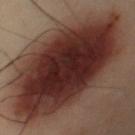The lesion is on the chest. A region of skin cropped from a whole-body photographic capture, roughly 15 mm wide. A male subject aged around 50. Longest diameter approximately 17.5 mm.